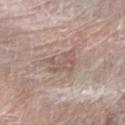Assessment:
Captured during whole-body skin photography for melanoma surveillance; the lesion was not biopsied.
Image and clinical context:
The lesion-visualizer software estimated a lesion area of about 6.5 mm², an outline eccentricity of about 0.45 (0 = round, 1 = elongated), and a shape-asymmetry score of about 0.4 (0 = symmetric). And it measured an automated nevus-likeness rating near 0 out of 100 and lesion-presence confidence of about 70/100. A male subject roughly 80 years of age. Imaged with white-light lighting. Located on the right forearm. This image is a 15 mm lesion crop taken from a total-body photograph. Measured at roughly 3 mm in maximum diameter.